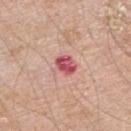Q: Was this lesion biopsied?
A: imaged on a skin check; not biopsied
Q: Illumination type?
A: white-light
Q: What did automated image analysis measure?
A: about 15 CIELAB-L* units darker than the surrounding skin and a lesion-to-skin contrast of about 10 (normalized; higher = more distinct); a border-irregularity rating of about 2/10, a within-lesion color-variation index near 5/10, and radial color variation of about 1.5; a nevus-likeness score of about 0/100 and a lesion-detection confidence of about 100/100
Q: What is the anatomic site?
A: the right upper arm
Q: What kind of image is this?
A: 15 mm crop, total-body photography
Q: What are the patient's age and sex?
A: male, about 60 years old
Q: Lesion size?
A: ~2.5 mm (longest diameter)